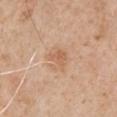Notes:
- workup: imaged on a skin check; not biopsied
- lighting: white-light
- body site: the left upper arm
- imaging modality: total-body-photography crop, ~15 mm field of view
- subject: male, roughly 60 years of age